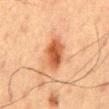Case summary:
– notes · imaged on a skin check; not biopsied
– subject · male, in their 60s
– lighting · cross-polarized illumination
– lesion size · ~4 mm (longest diameter)
– anatomic site · the back
– image · ~15 mm crop, total-body skin-cancer survey
– TBP lesion metrics · an area of roughly 10 mm²; a mean CIELAB color near L≈46 a*≈23 b*≈33, a lesion–skin lightness drop of about 11, and a normalized lesion–skin contrast near 9; a border-irregularity rating of about 1.5/10 and a within-lesion color-variation index near 5/10